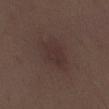Part of a total-body skin-imaging series; this lesion was reviewed on a skin check and was not flagged for biopsy.
The tile uses white-light illumination.
A region of skin cropped from a whole-body photographic capture, roughly 15 mm wide.
Measured at roughly 4.5 mm in maximum diameter.
The lesion is on the mid back.
The subject is a male aged 28–32.